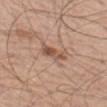Impression:
Recorded during total-body skin imaging; not selected for excision or biopsy.
Clinical summary:
This image is a 15 mm lesion crop taken from a total-body photograph. From the left thigh. About 3.5 mm across. The total-body-photography lesion software estimated roughly 11 lightness units darker than nearby skin. A male subject, aged approximately 70.